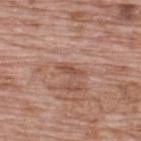<tbp_lesion>
<biopsy_status>not biopsied; imaged during a skin examination</biopsy_status>
<lighting>white-light</lighting>
<site>upper back</site>
<patient>
  <sex>male</sex>
  <age_approx>70</age_approx>
</patient>
<image>
  <source>total-body photography crop</source>
  <field_of_view_mm>15</field_of_view_mm>
</image>
<lesion_size>
  <long_diameter_mm_approx>3.5</long_diameter_mm_approx>
</lesion_size>
</tbp_lesion>